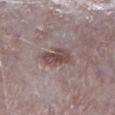Q: What kind of image is this?
A: total-body-photography crop, ~15 mm field of view
Q: How large is the lesion?
A: about 3.5 mm
Q: What did automated image analysis measure?
A: an eccentricity of roughly 0.75 and two-axis asymmetry of about 0.2
Q: What is the anatomic site?
A: the right lower leg
Q: Patient demographics?
A: male, in their mid-70s